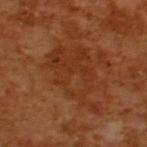{"automated_metrics": {"area_mm2_approx": 24.0, "shape_asymmetry": 0.35, "border_irregularity_0_10": 6.0, "color_variation_0_10": 4.5, "peripheral_color_asymmetry": 1.5}, "lesion_size": {"long_diameter_mm_approx": 8.0}, "image": {"source": "total-body photography crop", "field_of_view_mm": 15}, "patient": {"sex": "male", "age_approx": 65}, "lighting": "cross-polarized"}